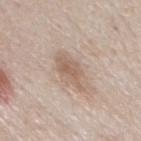{
  "biopsy_status": "not biopsied; imaged during a skin examination",
  "lesion_size": {
    "long_diameter_mm_approx": 5.0
  },
  "automated_metrics": {
    "border_irregularity_0_10": 3.5,
    "color_variation_0_10": 3.0
  },
  "lighting": "white-light",
  "image": {
    "source": "total-body photography crop",
    "field_of_view_mm": 15
  },
  "patient": {
    "sex": "male",
    "age_approx": 60
  },
  "site": "chest"
}